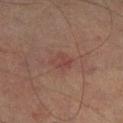site: the right lower leg
image: ~15 mm tile from a whole-body skin photo
subject: male, about 75 years old